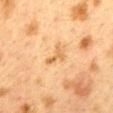Background: On the back. The lesion-visualizer software estimated an area of roughly 3 mm², an outline eccentricity of about 0.9 (0 = round, 1 = elongated), and two-axis asymmetry of about 0.5. And it measured an average lesion color of about L≈55 a*≈18 b*≈38 (CIELAB), a lesion–skin lightness drop of about 8, and a normalized lesion–skin contrast near 6.5. And it measured border irregularity of about 6 on a 0–10 scale, a within-lesion color-variation index near 0/10, and a peripheral color-asymmetry measure near 0. Approximately 3 mm at its widest. The tile uses cross-polarized illumination. A 15 mm crop from a total-body photograph taken for skin-cancer surveillance. A female patient, roughly 40 years of age.